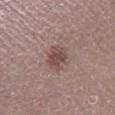Clinical impression: Imaged during a routine full-body skin examination; the lesion was not biopsied and no histopathology is available. Background: A 15 mm crop from a total-body photograph taken for skin-cancer surveillance. From the right lower leg. A male patient, in their mid- to late 60s.